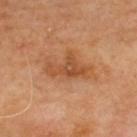  biopsy_status: not biopsied; imaged during a skin examination
  patient:
    sex: male
    age_approx: 70
  site: upper back
  image:
    source: total-body photography crop
    field_of_view_mm: 15
  lighting: cross-polarized
  lesion_size:
    long_diameter_mm_approx: 5.0